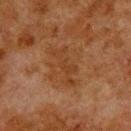workup: total-body-photography surveillance lesion; no biopsy | acquisition: total-body-photography crop, ~15 mm field of view | subject: male, about 80 years old | illumination: cross-polarized illumination | body site: the upper back.